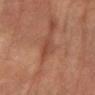Q: Was this lesion biopsied?
A: no biopsy performed (imaged during a skin exam)
Q: Illumination type?
A: cross-polarized
Q: How was this image acquired?
A: total-body-photography crop, ~15 mm field of view
Q: Who is the patient?
A: male, aged 83 or older
Q: What is the anatomic site?
A: the left forearm